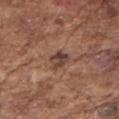Recorded during total-body skin imaging; not selected for excision or biopsy. A male patient aged 73–77. Located on the right upper arm. Cropped from a total-body skin-imaging series; the visible field is about 15 mm. Longest diameter approximately 2.5 mm.